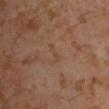Part of a total-body skin-imaging series; this lesion was reviewed on a skin check and was not flagged for biopsy.